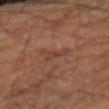anatomic site = the right upper arm | automated lesion analysis = a mean CIELAB color near L≈39 a*≈19 b*≈26 and a lesion–skin lightness drop of about 5; a nevus-likeness score of about 0/100 and a lesion-detection confidence of about 100/100 | imaging modality = ~15 mm crop, total-body skin-cancer survey | lighting = cross-polarized illumination | subject = male, aged 58 to 62.